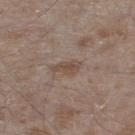The lesion was tiled from a total-body skin photograph and was not biopsied.
Approximately 3.5 mm at its widest.
Imaged with white-light lighting.
Cropped from a whole-body photographic skin survey; the tile spans about 15 mm.
Located on the left lower leg.
The subject is a male approximately 45 years of age.
Automated image analysis of the tile measured a lesion color around L≈47 a*≈15 b*≈24 in CIELAB, about 8 CIELAB-L* units darker than the surrounding skin, and a normalized lesion–skin contrast near 6.5. The software also gave border irregularity of about 3.5 on a 0–10 scale and a peripheral color-asymmetry measure near 0.5.